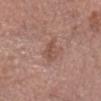Part of a total-body skin-imaging series; this lesion was reviewed on a skin check and was not flagged for biopsy.
Imaged with white-light lighting.
A male subject approximately 60 years of age.
The lesion is on the head or neck.
Automated image analysis of the tile measured a footprint of about 3 mm² and an outline eccentricity of about 0.95 (0 = round, 1 = elongated). The software also gave a lesion color around L≈50 a*≈21 b*≈26 in CIELAB and about 8 CIELAB-L* units darker than the surrounding skin. The analysis additionally found border irregularity of about 4.5 on a 0–10 scale, internal color variation of about 0 on a 0–10 scale, and a peripheral color-asymmetry measure near 0.
Longest diameter approximately 3 mm.
A region of skin cropped from a whole-body photographic capture, roughly 15 mm wide.